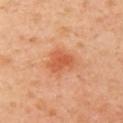| key | value |
|---|---|
| workup | no biopsy performed (imaged during a skin exam) |
| size | ≈3.5 mm |
| subject | female, aged approximately 40 |
| location | the left upper arm |
| image | total-body-photography crop, ~15 mm field of view |